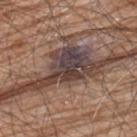<case>
<biopsy_status>not biopsied; imaged during a skin examination</biopsy_status>
<lighting>white-light</lighting>
<image>
  <source>total-body photography crop</source>
  <field_of_view_mm>15</field_of_view_mm>
</image>
<patient>
  <sex>male</sex>
  <age_approx>80</age_approx>
</patient>
<site>back</site>
<automated_metrics>
  <border_irregularity_0_10>8.0</border_irregularity_0_10>
  <lesion_detection_confidence_0_100>5</lesion_detection_confidence_0_100>
</automated_metrics>
</case>